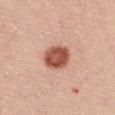{"image": {"source": "total-body photography crop", "field_of_view_mm": 15}, "lighting": "white-light", "lesion_size": {"long_diameter_mm_approx": 3.5}, "patient": {"sex": "female", "age_approx": 25}, "automated_metrics": {"border_irregularity_0_10": 1.0, "peripheral_color_asymmetry": 1.5, "nevus_likeness_0_100": 100, "lesion_detection_confidence_0_100": 100}, "site": "front of the torso"}